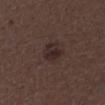Part of a total-body skin-imaging series; this lesion was reviewed on a skin check and was not flagged for biopsy.
From the right lower leg.
Measured at roughly 3 mm in maximum diameter.
A male patient, approximately 50 years of age.
Imaged with white-light lighting.
A region of skin cropped from a whole-body photographic capture, roughly 15 mm wide.
An algorithmic analysis of the crop reported a footprint of about 5.5 mm², a shape eccentricity near 0.6, and two-axis asymmetry of about 0.3. It also reported a lesion–skin lightness drop of about 7 and a lesion-to-skin contrast of about 8 (normalized; higher = more distinct). The analysis additionally found a border-irregularity rating of about 2.5/10 and a color-variation rating of about 3/10.